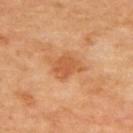Q: Is there a histopathology result?
A: imaged on a skin check; not biopsied
Q: What is the imaging modality?
A: ~15 mm crop, total-body skin-cancer survey
Q: What are the patient's age and sex?
A: in their mid-60s
Q: Lesion location?
A: the back
Q: Automated lesion metrics?
A: a lesion color around L≈56 a*≈26 b*≈40 in CIELAB, a lesion–skin lightness drop of about 9, and a lesion-to-skin contrast of about 6.5 (normalized; higher = more distinct)